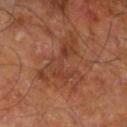This lesion was catalogued during total-body skin photography and was not selected for biopsy.
The patient is a male aged 58–62.
This image is a 15 mm lesion crop taken from a total-body photograph.
Captured under cross-polarized illumination.
Longest diameter approximately 8 mm.
Located on the leg.